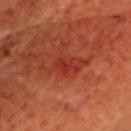| key | value |
|---|---|
| workup | no biopsy performed (imaged during a skin exam) |
| anatomic site | the head or neck |
| acquisition | ~15 mm tile from a whole-body skin photo |
| subject | female, aged 78 to 82 |